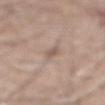Acquisition and patient details: A 15 mm close-up extracted from a 3D total-body photography capture. The patient is a male in their 60s. From the mid back.